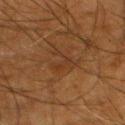Clinical impression: This lesion was catalogued during total-body skin photography and was not selected for biopsy. Acquisition and patient details: On the left lower leg. A male patient in their mid-60s. A roughly 15 mm field-of-view crop from a total-body skin photograph. The lesion's longest dimension is about 3 mm.